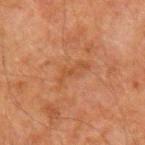Findings:
* workup: imaged on a skin check; not biopsied
* imaging modality: total-body-photography crop, ~15 mm field of view
* subject: male, in their 60s
* anatomic site: the arm